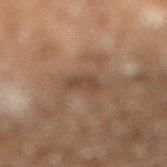Assessment:
No biopsy was performed on this lesion — it was imaged during a full skin examination and was not determined to be concerning.
Context:
The subject is a male approximately 60 years of age. A roughly 15 mm field-of-view crop from a total-body skin photograph. This is a cross-polarized tile. From the right lower leg. Measured at roughly 2.5 mm in maximum diameter.